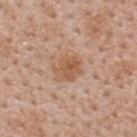biopsy_status: not biopsied; imaged during a skin examination
site: upper back
patient:
  sex: male
  age_approx: 40
image:
  source: total-body photography crop
  field_of_view_mm: 15